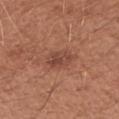<tbp_lesion>
  <patient>
    <sex>female</sex>
    <age_approx>40</age_approx>
  </patient>
  <site>left forearm</site>
  <image>
    <source>total-body photography crop</source>
    <field_of_view_mm>15</field_of_view_mm>
  </image>
</tbp_lesion>